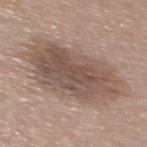The lesion was tiled from a total-body skin photograph and was not biopsied. This is a white-light tile. Longest diameter approximately 10 mm. The lesion-visualizer software estimated a lesion–skin lightness drop of about 11 and a lesion-to-skin contrast of about 7.5 (normalized; higher = more distinct). The analysis additionally found a color-variation rating of about 5/10 and radial color variation of about 1.5. The software also gave an automated nevus-likeness rating near 20 out of 100 and a detector confidence of about 100 out of 100 that the crop contains a lesion. The subject is a male about 55 years old. From the mid back. Cropped from a whole-body photographic skin survey; the tile spans about 15 mm.